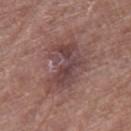Q: Is there a histopathology result?
A: total-body-photography surveillance lesion; no biopsy
Q: How was this image acquired?
A: 15 mm crop, total-body photography
Q: Lesion location?
A: the right thigh
Q: Patient demographics?
A: female, in their mid-70s